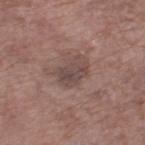Case summary:
• workup — catalogued during a skin exam; not biopsied
• tile lighting — white-light
• diameter — about 3.5 mm
• image source — 15 mm crop, total-body photography
• patient — male, aged 68 to 72
• location — the right lower leg